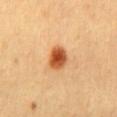Recorded during total-body skin imaging; not selected for excision or biopsy. This image is a 15 mm lesion crop taken from a total-body photograph. The subject is a female aged 43 to 47. On the mid back. The lesion's longest dimension is about 3 mm. Imaged with cross-polarized lighting.